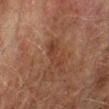biopsy_status: not biopsied; imaged during a skin examination
lesion_size:
  long_diameter_mm_approx: 4.0
lighting: cross-polarized
site: left lower leg
image:
  source: total-body photography crop
  field_of_view_mm: 15
patient:
  sex: male
  age_approx: 75